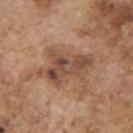{"biopsy_status": "not biopsied; imaged during a skin examination", "lesion_size": {"long_diameter_mm_approx": 6.5}, "image": {"source": "total-body photography crop", "field_of_view_mm": 15}, "site": "right upper arm", "patient": {"sex": "male", "age_approx": 75}}